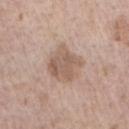<lesion>
<biopsy_status>not biopsied; imaged during a skin examination</biopsy_status>
<lesion_size>
  <long_diameter_mm_approx>4.5</long_diameter_mm_approx>
</lesion_size>
<patient>
  <sex>male</sex>
  <age_approx>60</age_approx>
</patient>
<image>
  <source>total-body photography crop</source>
  <field_of_view_mm>15</field_of_view_mm>
</image>
<site>arm</site>
</lesion>